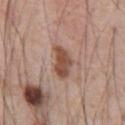Captured during whole-body skin photography for melanoma surveillance; the lesion was not biopsied. A male patient, roughly 55 years of age. Imaged with white-light lighting. A lesion tile, about 15 mm wide, cut from a 3D total-body photograph. The recorded lesion diameter is about 4 mm. The lesion is on the front of the torso.